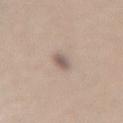follow-up: catalogued during a skin exam; not biopsied.